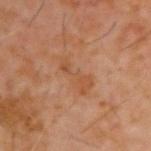notes: imaged on a skin check; not biopsied | body site: the upper back | lighting: cross-polarized | TBP lesion metrics: an outline eccentricity of about 0.9 (0 = round, 1 = elongated) and a symmetry-axis asymmetry near 0.45; roughly 5 lightness units darker than nearby skin and a normalized border contrast of about 5; border irregularity of about 6 on a 0–10 scale, internal color variation of about 2 on a 0–10 scale, and radial color variation of about 0.5 | lesion diameter: ≈5 mm | subject: male, aged 58 to 62 | imaging modality: ~15 mm tile from a whole-body skin photo.